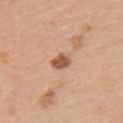Q: Was this lesion biopsied?
A: catalogued during a skin exam; not biopsied
Q: Who is the patient?
A: female, roughly 60 years of age
Q: Automated lesion metrics?
A: a lesion area of about 4 mm²; a lesion-detection confidence of about 100/100
Q: Lesion location?
A: the left upper arm
Q: How was this image acquired?
A: total-body-photography crop, ~15 mm field of view
Q: How large is the lesion?
A: about 2.5 mm